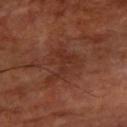Findings:
– follow-up — total-body-photography surveillance lesion; no biopsy
– anatomic site — the left forearm
– acquisition — total-body-photography crop, ~15 mm field of view
– subject — in their mid- to late 60s
– lighting — cross-polarized illumination
– diameter — ≈4 mm